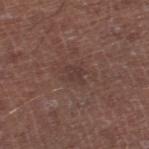The lesion was photographed on a routine skin check and not biopsied; there is no pathology result.
Captured under white-light illumination.
Cropped from a whole-body photographic skin survey; the tile spans about 15 mm.
The lesion's longest dimension is about 2.5 mm.
The lesion is on the leg.
The total-body-photography lesion software estimated an area of roughly 3.5 mm², an eccentricity of roughly 0.6, and two-axis asymmetry of about 0.35. And it measured a mean CIELAB color near L≈36 a*≈18 b*≈20 and a normalized lesion–skin contrast near 5.5. It also reported a color-variation rating of about 1/10.
A male patient in their mid-60s.